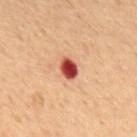Impression: The lesion was photographed on a routine skin check and not biopsied; there is no pathology result.